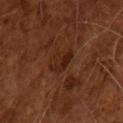Imaged during a routine full-body skin examination; the lesion was not biopsied and no histopathology is available.
A male patient aged approximately 65.
The recorded lesion diameter is about 3 mm.
A roughly 15 mm field-of-view crop from a total-body skin photograph.